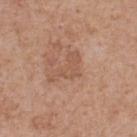Notes:
- subject: male, roughly 60 years of age
- location: the upper back
- image-analysis metrics: a footprint of about 6.5 mm², a shape eccentricity near 0.85, and a symmetry-axis asymmetry near 0.7; a mean CIELAB color near L≈54 a*≈21 b*≈30 and a lesion–skin lightness drop of about 6; a color-variation rating of about 1/10
- image: ~15 mm crop, total-body skin-cancer survey